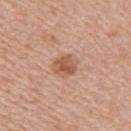notes: imaged on a skin check; not biopsied | image-analysis metrics: a lesion color around L≈56 a*≈22 b*≈31 in CIELAB, a lesion–skin lightness drop of about 10, and a lesion-to-skin contrast of about 7 (normalized; higher = more distinct); a border-irregularity index near 2/10, internal color variation of about 4 on a 0–10 scale, and peripheral color asymmetry of about 1.5; a detector confidence of about 100 out of 100 that the crop contains a lesion | image: ~15 mm crop, total-body skin-cancer survey | size: about 3 mm | illumination: white-light illumination | body site: the right upper arm | patient: female, aged around 70.